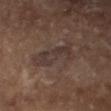Clinical impression: Imaged during a routine full-body skin examination; the lesion was not biopsied and no histopathology is available. Background: The recorded lesion diameter is about 4.5 mm. A roughly 15 mm field-of-view crop from a total-body skin photograph. From the left forearm. Automated image analysis of the tile measured border irregularity of about 5 on a 0–10 scale and radial color variation of about 1. The analysis additionally found a nevus-likeness score of about 0/100 and a detector confidence of about 90 out of 100 that the crop contains a lesion. A female patient, roughly 70 years of age. This is a cross-polarized tile.